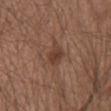Case summary:
- biopsy status · catalogued during a skin exam; not biopsied
- site · the abdomen
- diameter · about 2.5 mm
- patient · male, aged approximately 65
- imaging modality · 15 mm crop, total-body photography
- illumination · white-light illumination